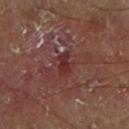Part of a total-body skin-imaging series; this lesion was reviewed on a skin check and was not flagged for biopsy. The patient is a male approximately 70 years of age. A roughly 15 mm field-of-view crop from a total-body skin photograph. On the right lower leg. Automated tile analysis of the lesion measured a footprint of about 4 mm², an outline eccentricity of about 0.8 (0 = round, 1 = elongated), and a shape-asymmetry score of about 0.3 (0 = symmetric). It also reported a mean CIELAB color near L≈30 a*≈25 b*≈21 and about 7 CIELAB-L* units darker than the surrounding skin. The software also gave an automated nevus-likeness rating near 0 out of 100 and lesion-presence confidence of about 80/100. Approximately 2.5 mm at its widest.